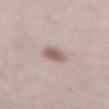Case summary:
• follow-up: catalogued during a skin exam; not biopsied
• imaging modality: total-body-photography crop, ~15 mm field of view
• location: the lower back
• patient: female, aged approximately 40